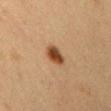  biopsy_status: not biopsied; imaged during a skin examination
  lesion_size:
    long_diameter_mm_approx: 2.5
  lighting: cross-polarized
  image:
    source: total-body photography crop
    field_of_view_mm: 15
  automated_metrics:
    shape_asymmetry: 0.2
    vs_skin_darker_L: 14.0
    vs_skin_contrast_norm: 11.0
    border_irregularity_0_10: 2.0
    color_variation_0_10: 5.0
    peripheral_color_asymmetry: 1.5
    nevus_likeness_0_100: 100
  patient:
    sex: female
    age_approx: 30
  site: right upper arm